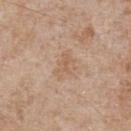follow-up=no biopsy performed (imaged during a skin exam); tile lighting=white-light illumination; imaging modality=~15 mm tile from a whole-body skin photo; body site=the front of the torso; subject=male, aged around 65; diameter=~3.5 mm (longest diameter).